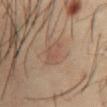Assessment:
Part of a total-body skin-imaging series; this lesion was reviewed on a skin check and was not flagged for biopsy.
Context:
A 15 mm close-up extracted from a 3D total-body photography capture. The lesion is on the abdomen. The tile uses cross-polarized illumination. A male subject in their 40s.